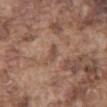Q: Was this lesion biopsied?
A: imaged on a skin check; not biopsied
Q: What are the patient's age and sex?
A: male, in their mid- to late 70s
Q: Illumination type?
A: white-light illumination
Q: Where on the body is the lesion?
A: the chest
Q: What did automated image analysis measure?
A: an area of roughly 2.5 mm², an outline eccentricity of about 0.9 (0 = round, 1 = elongated), and two-axis asymmetry of about 0.25; a lesion color around L≈48 a*≈18 b*≈27 in CIELAB, a lesion–skin lightness drop of about 7, and a normalized border contrast of about 5.5; a border-irregularity index near 3/10, a within-lesion color-variation index near 0/10, and radial color variation of about 0
Q: What kind of image is this?
A: total-body-photography crop, ~15 mm field of view